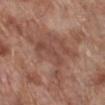<lesion>
  <site>right lower leg</site>
  <lighting>white-light</lighting>
  <image>
    <source>total-body photography crop</source>
    <field_of_view_mm>15</field_of_view_mm>
  </image>
  <automated_metrics>
    <cielab_L>48</cielab_L>
    <cielab_a>21</cielab_a>
    <cielab_b>26</cielab_b>
    <vs_skin_darker_L>7.0</vs_skin_darker_L>
    <vs_skin_contrast_norm>5.5</vs_skin_contrast_norm>
  </automated_metrics>
  <patient>
    <sex>male</sex>
    <age_approx>70</age_approx>
  </patient>
  <lesion_size>
    <long_diameter_mm_approx>7.5</long_diameter_mm_approx>
  </lesion_size>
</lesion>